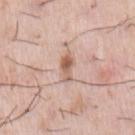Recorded during total-body skin imaging; not selected for excision or biopsy. A male patient, roughly 75 years of age. A region of skin cropped from a whole-body photographic capture, roughly 15 mm wide. Captured under white-light illumination. The recorded lesion diameter is about 3 mm. Located on the chest.